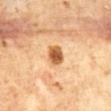Notes:
- workup · no biopsy performed (imaged during a skin exam)
- subject · male, in their mid-60s
- anatomic site · the abdomen
- acquisition · ~15 mm tile from a whole-body skin photo